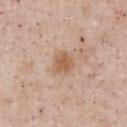| field | value |
|---|---|
| workup | catalogued during a skin exam; not biopsied |
| lighting | white-light illumination |
| anatomic site | the chest |
| patient | male, approximately 55 years of age |
| image source | ~15 mm crop, total-body skin-cancer survey |
| lesion size | ~2.5 mm (longest diameter) |